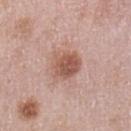<lesion>
<biopsy_status>not biopsied; imaged during a skin examination</biopsy_status>
<image>
  <source>total-body photography crop</source>
  <field_of_view_mm>15</field_of_view_mm>
</image>
<site>left upper arm</site>
<patient>
  <sex>female</sex>
  <age_approx>50</age_approx>
</patient>
<lighting>white-light</lighting>
</lesion>